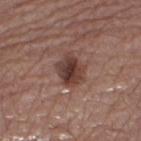Assessment:
The lesion was photographed on a routine skin check and not biopsied; there is no pathology result.
Background:
The total-body-photography lesion software estimated an area of roughly 9 mm², an eccentricity of roughly 0.6, and a shape-asymmetry score of about 0.25 (0 = symmetric). And it measured an average lesion color of about L≈39 a*≈20 b*≈23 (CIELAB), about 12 CIELAB-L* units darker than the surrounding skin, and a normalized lesion–skin contrast near 9.5. The analysis additionally found a border-irregularity rating of about 2.5/10, a color-variation rating of about 5.5/10, and peripheral color asymmetry of about 1.5. A male subject aged 63 to 67. Captured under white-light illumination. A region of skin cropped from a whole-body photographic capture, roughly 15 mm wide. About 4 mm across. Located on the left thigh.